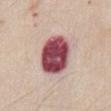Findings:
* workup — imaged on a skin check; not biopsied
* lighting — white-light illumination
* acquisition — total-body-photography crop, ~15 mm field of view
* site — the chest
* automated metrics — a lesion area of about 18 mm² and a shape eccentricity near 0.65; a border-irregularity index near 1/10, internal color variation of about 7 on a 0–10 scale, and radial color variation of about 2
* subject — male, about 45 years old
* lesion size — ~5.5 mm (longest diameter)